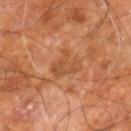Assessment:
Captured during whole-body skin photography for melanoma surveillance; the lesion was not biopsied.
Background:
The lesion is on the right leg. A roughly 15 mm field-of-view crop from a total-body skin photograph. A male patient, about 60 years old. Longest diameter approximately 4 mm.